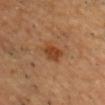Impression:
No biopsy was performed on this lesion — it was imaged during a full skin examination and was not determined to be concerning.
Clinical summary:
The lesion is on the head or neck. The patient is a male about 45 years old. A lesion tile, about 15 mm wide, cut from a 3D total-body photograph.